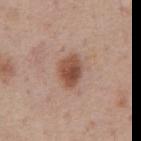Imaged during a routine full-body skin examination; the lesion was not biopsied and no histopathology is available.
A male patient approximately 60 years of age.
On the chest.
A roughly 15 mm field-of-view crop from a total-body skin photograph.